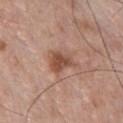{"biopsy_status": "not biopsied; imaged during a skin examination", "patient": {"sex": "male", "age_approx": 55}, "site": "chest", "image": {"source": "total-body photography crop", "field_of_view_mm": 15}, "automated_metrics": {"vs_skin_darker_L": 11.0, "border_irregularity_0_10": 3.5, "color_variation_0_10": 3.5, "peripheral_color_asymmetry": 1.0}, "lighting": "white-light"}